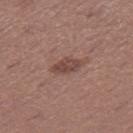Q: Was a biopsy performed?
A: no biopsy performed (imaged during a skin exam)
Q: Illumination type?
A: white-light
Q: Automated lesion metrics?
A: an area of roughly 5 mm², an eccentricity of roughly 0.85, and a symmetry-axis asymmetry near 0.25; a lesion color around L≈45 a*≈20 b*≈23 in CIELAB
Q: Patient demographics?
A: female, aged 23 to 27
Q: Lesion location?
A: the right thigh
Q: What kind of image is this?
A: ~15 mm tile from a whole-body skin photo
Q: What is the lesion's diameter?
A: ≈3.5 mm